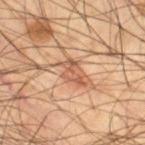Notes:
- biopsy status · total-body-photography surveillance lesion; no biopsy
- automated lesion analysis · a footprint of about 4.5 mm², an outline eccentricity of about 0.85 (0 = round, 1 = elongated), and two-axis asymmetry of about 0.5; a mean CIELAB color near L≈52 a*≈22 b*≈31 and a lesion-to-skin contrast of about 7 (normalized; higher = more distinct); a nevus-likeness score of about 45/100
- body site · the right thigh
- image source · ~15 mm crop, total-body skin-cancer survey
- illumination · cross-polarized
- diameter · ~3.5 mm (longest diameter)
- patient · male, roughly 55 years of age